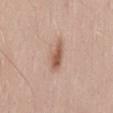workup: total-body-photography surveillance lesion; no biopsy | lesion size: ≈4 mm | image source: ~15 mm tile from a whole-body skin photo | patient: male, aged around 50 | TBP lesion metrics: a lesion area of about 6 mm² and two-axis asymmetry of about 0.2; a classifier nevus-likeness of about 90/100 and a detector confidence of about 100 out of 100 that the crop contains a lesion | site: the lower back.